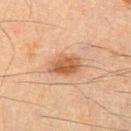{
  "biopsy_status": "not biopsied; imaged during a skin examination",
  "lighting": "cross-polarized",
  "site": "leg",
  "image": {
    "source": "total-body photography crop",
    "field_of_view_mm": 15
  },
  "automated_metrics": {
    "area_mm2_approx": 9.0,
    "eccentricity": 0.8,
    "shape_asymmetry": 0.2
  },
  "patient": {
    "sex": "male",
    "age_approx": 65
  },
  "lesion_size": {
    "long_diameter_mm_approx": 4.0
  }
}